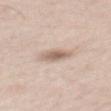biopsy_status: not biopsied; imaged during a skin examination
automated_metrics:
  cielab_L: 63
  cielab_a: 15
  cielab_b: 26
  vs_skin_contrast_norm: 7.5
  nevus_likeness_0_100: 60
lesion_size:
  long_diameter_mm_approx: 3.0
lighting: white-light
image:
  source: total-body photography crop
  field_of_view_mm: 15
patient:
  sex: male
  age_approx: 40
site: mid back